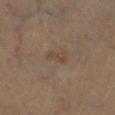workup=imaged on a skin check; not biopsied
image source=~15 mm tile from a whole-body skin photo
patient=male, about 45 years old
body site=the left lower leg